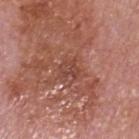Assessment: Imaged during a routine full-body skin examination; the lesion was not biopsied and no histopathology is available. Clinical summary: This is a white-light tile. About 2 mm across. A lesion tile, about 15 mm wide, cut from a 3D total-body photograph. The lesion is located on the head or neck. The subject is a male aged around 80.